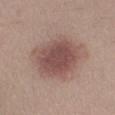<lesion>
<biopsy_status>not biopsied; imaged during a skin examination</biopsy_status>
<lesion_size>
  <long_diameter_mm_approx>7.0</long_diameter_mm_approx>
</lesion_size>
<lighting>white-light</lighting>
<patient>
  <sex>female</sex>
  <age_approx>25</age_approx>
</patient>
<automated_metrics>
  <cielab_L>51</cielab_L>
  <cielab_a>19</cielab_a>
  <cielab_b>22</cielab_b>
  <vs_skin_contrast_norm>8.5</vs_skin_contrast_norm>
  <nevus_likeness_0_100>95</nevus_likeness_0_100>
  <lesion_detection_confidence_0_100>100</lesion_detection_confidence_0_100>
</automated_metrics>
<site>left lower leg</site>
<image>
  <source>total-body photography crop</source>
  <field_of_view_mm>15</field_of_view_mm>
</image>
</lesion>